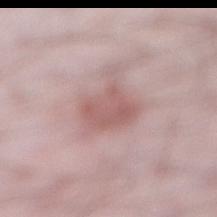The lesion was tiled from a total-body skin photograph and was not biopsied. Cropped from a total-body skin-imaging series; the visible field is about 15 mm. On the right lower leg. Automated tile analysis of the lesion measured an area of roughly 13 mm² and a symmetry-axis asymmetry near 0.35. The software also gave a classifier nevus-likeness of about 60/100 and lesion-presence confidence of about 100/100. The recorded lesion diameter is about 5 mm. The tile uses white-light illumination. The subject is a male aged 23 to 27.